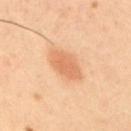The subject is a male roughly 40 years of age.
The lesion is located on the upper back.
Imaged with cross-polarized lighting.
Longest diameter approximately 5 mm.
A region of skin cropped from a whole-body photographic capture, roughly 15 mm wide.